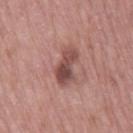Captured during whole-body skin photography for melanoma surveillance; the lesion was not biopsied. The lesion is on the right thigh. Automated image analysis of the tile measured a nevus-likeness score of about 40/100. Approximately 5 mm at its widest. A 15 mm close-up tile from a total-body photography series done for melanoma screening. A female subject about 65 years old. This is a white-light tile.